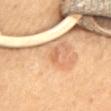lesion diameter — ~2 mm (longest diameter)
body site — the mid back
subject — female, aged 63–67
lighting — cross-polarized
acquisition — 15 mm crop, total-body photography